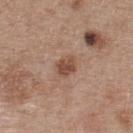tile lighting: white-light | body site: the upper back | TBP lesion metrics: an area of roughly 4.5 mm² and two-axis asymmetry of about 0.2; border irregularity of about 1.5 on a 0–10 scale and a color-variation rating of about 3/10 | image source: 15 mm crop, total-body photography | patient: female, in their 40s | diameter: about 2.5 mm.